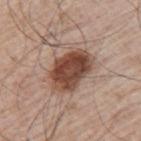Recorded during total-body skin imaging; not selected for excision or biopsy. The tile uses white-light illumination. Automated image analysis of the tile measured an eccentricity of roughly 0.75. The analysis additionally found an automated nevus-likeness rating near 70 out of 100 and lesion-presence confidence of about 100/100. A roughly 15 mm field-of-view crop from a total-body skin photograph. A male subject in their mid-60s. The lesion is on the left upper arm.